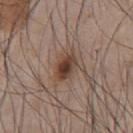image:
  source: total-body photography crop
  field_of_view_mm: 15
patient:
  sex: male
  age_approx: 45
site: chest